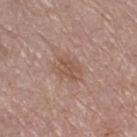biopsy status = no biopsy performed (imaged during a skin exam); lighting = white-light illumination; imaging modality = total-body-photography crop, ~15 mm field of view; patient = female, aged around 55; anatomic site = the right thigh; lesion diameter = about 3.5 mm.